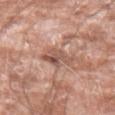Imaged during a routine full-body skin examination; the lesion was not biopsied and no histopathology is available. A male patient, aged 58 to 62. From the left forearm. Captured under white-light illumination. A lesion tile, about 15 mm wide, cut from a 3D total-body photograph.